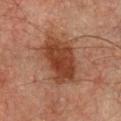notes=no biopsy performed (imaged during a skin exam)